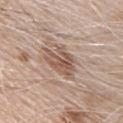follow-up: total-body-photography surveillance lesion; no biopsy | subject: male, aged 68–72 | image-analysis metrics: a shape eccentricity near 0.8 and two-axis asymmetry of about 0.35; lesion-presence confidence of about 100/100 | lesion size: about 5 mm | imaging modality: ~15 mm crop, total-body skin-cancer survey | site: the chest.